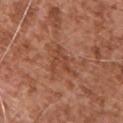Imaged during a routine full-body skin examination; the lesion was not biopsied and no histopathology is available. The total-body-photography lesion software estimated border irregularity of about 8.5 on a 0–10 scale, a color-variation rating of about 1.5/10, and a peripheral color-asymmetry measure near 0.5. On the upper back. A male patient aged 73 to 77. A region of skin cropped from a whole-body photographic capture, roughly 15 mm wide.